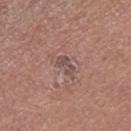biopsy status: imaged on a skin check; not biopsied | site: the right thigh | subject: female, in their 50s | lesion diameter: ~2.5 mm (longest diameter) | TBP lesion metrics: a footprint of about 3 mm² and a shape eccentricity near 0.85; an average lesion color of about L≈49 a*≈18 b*≈21 (CIELAB) and roughly 8 lightness units darker than nearby skin; a border-irregularity rating of about 3/10, a color-variation rating of about 1.5/10, and a peripheral color-asymmetry measure near 0.5 | imaging modality: total-body-photography crop, ~15 mm field of view | illumination: white-light.